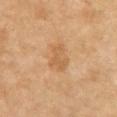biopsy status=no biopsy performed (imaged during a skin exam)
automated lesion analysis=a lesion color around L≈51 a*≈18 b*≈35 in CIELAB and a normalized lesion–skin contrast near 5.5; a nevus-likeness score of about 0/100 and lesion-presence confidence of about 100/100
subject=female, aged around 60
anatomic site=the chest
size=≈3.5 mm
imaging modality=15 mm crop, total-body photography